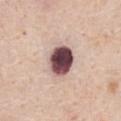Captured during whole-body skin photography for melanoma surveillance; the lesion was not biopsied. Longest diameter approximately 4 mm. The patient is a male aged 58 to 62. Imaged with white-light lighting. A region of skin cropped from a whole-body photographic capture, roughly 15 mm wide. An algorithmic analysis of the crop reported a shape-asymmetry score of about 0.1 (0 = symmetric). And it measured about 26 CIELAB-L* units darker than the surrounding skin and a normalized border contrast of about 17. It also reported internal color variation of about 9 on a 0–10 scale and radial color variation of about 2.5. The lesion is located on the chest.